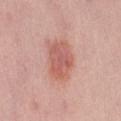follow-up=catalogued during a skin exam; not biopsied | image source=total-body-photography crop, ~15 mm field of view | location=the lower back | tile lighting=white-light | diameter=about 4.5 mm | patient=male, approximately 40 years of age.